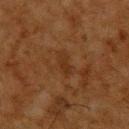{
  "biopsy_status": "not biopsied; imaged during a skin examination",
  "site": "back",
  "lesion_size": {
    "long_diameter_mm_approx": 3.0
  },
  "lighting": "cross-polarized",
  "patient": {
    "sex": "male",
    "age_approx": 60
  },
  "image": {
    "source": "total-body photography crop",
    "field_of_view_mm": 15
  }
}